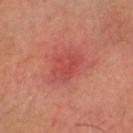Image and clinical context: A 15 mm close-up extracted from a 3D total-body photography capture. Longest diameter approximately 4 mm. On the head or neck. A male subject aged 68–72. The total-body-photography lesion software estimated an average lesion color of about L≈39 a*≈29 b*≈24 (CIELAB), a lesion–skin lightness drop of about 6, and a normalized lesion–skin contrast near 5. And it measured border irregularity of about 2.5 on a 0–10 scale, internal color variation of about 2.5 on a 0–10 scale, and a peripheral color-asymmetry measure near 1. Captured under cross-polarized illumination.